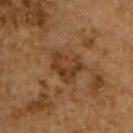Part of a total-body skin-imaging series; this lesion was reviewed on a skin check and was not flagged for biopsy.
The tile uses cross-polarized illumination.
Longest diameter approximately 4.5 mm.
Cropped from a total-body skin-imaging series; the visible field is about 15 mm.
The total-body-photography lesion software estimated a lesion area of about 10 mm², an eccentricity of roughly 0.65, and two-axis asymmetry of about 0.3. And it measured an average lesion color of about L≈34 a*≈18 b*≈31 (CIELAB), roughly 7 lightness units darker than nearby skin, and a lesion-to-skin contrast of about 7 (normalized; higher = more distinct). The software also gave a within-lesion color-variation index near 4/10 and radial color variation of about 1.5. And it measured lesion-presence confidence of about 85/100.
The subject is a male aged 63 to 67.
On the upper back.